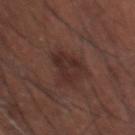biopsy_status: not biopsied; imaged during a skin examination
site: head or neck
image:
  source: total-body photography crop
  field_of_view_mm: 15
patient:
  sex: male
  age_approx: 35